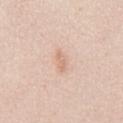| key | value |
|---|---|
| follow-up | total-body-photography surveillance lesion; no biopsy |